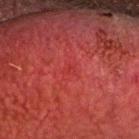follow-up: total-body-photography surveillance lesion; no biopsy
image source: 15 mm crop, total-body photography
diameter: about 1.5 mm
subject: male, in their 60s
tile lighting: cross-polarized illumination
body site: the head or neck
image-analysis metrics: a mean CIELAB color near L≈30 a*≈36 b*≈26 and a lesion–skin lightness drop of about 3; lesion-presence confidence of about 40/100